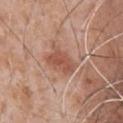follow-up: no biopsy performed (imaged during a skin exam) | illumination: white-light illumination | body site: the chest | subject: male, aged 63–67 | imaging modality: 15 mm crop, total-body photography | image-analysis metrics: a lesion color around L≈53 a*≈24 b*≈29 in CIELAB, about 9 CIELAB-L* units darker than the surrounding skin, and a normalized border contrast of about 7.